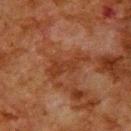Assessment: The lesion was tiled from a total-body skin photograph and was not biopsied. Background: A 15 mm close-up extracted from a 3D total-body photography capture. About 5.5 mm across. The subject is a male roughly 80 years of age. Located on the back.